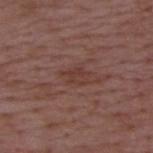No biopsy was performed on this lesion — it was imaged during a full skin examination and was not determined to be concerning.
A male patient aged approximately 50.
The lesion is on the upper back.
About 3 mm across.
A 15 mm close-up tile from a total-body photography series done for melanoma screening.